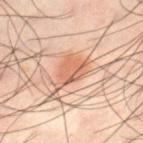Findings:
- workup · no biopsy performed (imaged during a skin exam)
- diameter · about 5 mm
- site · the left thigh
- image-analysis metrics · a footprint of about 9.5 mm² and an eccentricity of roughly 0.75; a mean CIELAB color near L≈63 a*≈24 b*≈34, roughly 10 lightness units darker than nearby skin, and a lesion-to-skin contrast of about 7 (normalized; higher = more distinct); a classifier nevus-likeness of about 100/100 and a lesion-detection confidence of about 100/100
- subject · male, about 40 years old
- image source · ~15 mm tile from a whole-body skin photo
- tile lighting · cross-polarized